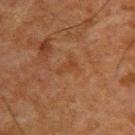biopsy status — catalogued during a skin exam; not biopsied
lesion diameter — ~2.5 mm (longest diameter)
image — total-body-photography crop, ~15 mm field of view
subject — male, about 65 years old
lighting — cross-polarized illumination
body site — the upper back